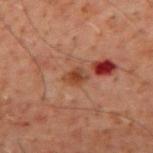Findings:
• biopsy status: no biopsy performed (imaged during a skin exam)
• site: the mid back
• acquisition: ~15 mm crop, total-body skin-cancer survey
• subject: male, approximately 60 years of age